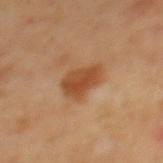Clinical summary:
Approximately 4 mm at its widest. A roughly 15 mm field-of-view crop from a total-body skin photograph. A male subject aged 63–67. The lesion is located on the mid back.